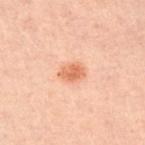<case>
  <biopsy_status>not biopsied; imaged during a skin examination</biopsy_status>
  <site>chest</site>
  <image>
    <source>total-body photography crop</source>
    <field_of_view_mm>15</field_of_view_mm>
  </image>
  <lighting>cross-polarized</lighting>
  <patient>
    <sex>male</sex>
    <age_approx>50</age_approx>
  </patient>
</case>